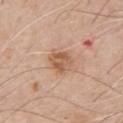Q: Was this lesion biopsied?
A: total-body-photography surveillance lesion; no biopsy
Q: What are the patient's age and sex?
A: male, about 70 years old
Q: How was this image acquired?
A: ~15 mm crop, total-body skin-cancer survey
Q: Automated lesion metrics?
A: radial color variation of about 1.5; a classifier nevus-likeness of about 30/100 and a lesion-detection confidence of about 100/100
Q: Illumination type?
A: white-light
Q: Where on the body is the lesion?
A: the chest
Q: What is the lesion's diameter?
A: ~3 mm (longest diameter)